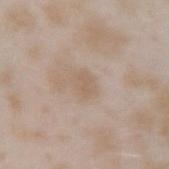Imaged during a routine full-body skin examination; the lesion was not biopsied and no histopathology is available.